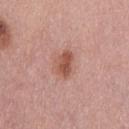Case summary:
* follow-up: total-body-photography surveillance lesion; no biopsy
* acquisition: ~15 mm crop, total-body skin-cancer survey
* subject: male, roughly 30 years of age